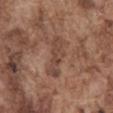Case summary:
– notes — catalogued during a skin exam; not biopsied
– image — ~15 mm tile from a whole-body skin photo
– subject — male, aged approximately 75
– lighting — white-light illumination
– anatomic site — the abdomen